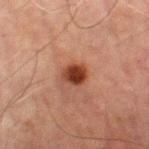workup=imaged on a skin check; not biopsied | subject=male, approximately 70 years of age | TBP lesion metrics=a border-irregularity rating of about 1.5/10, internal color variation of about 3.5 on a 0–10 scale, and peripheral color asymmetry of about 1 | diameter=≈2.5 mm | site=the leg | illumination=cross-polarized illumination | image=~15 mm tile from a whole-body skin photo.